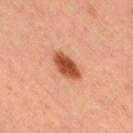Clinical impression: Captured during whole-body skin photography for melanoma surveillance; the lesion was not biopsied. Acquisition and patient details: The total-body-photography lesion software estimated a border-irregularity rating of about 2/10 and radial color variation of about 1. It also reported a classifier nevus-likeness of about 100/100 and a detector confidence of about 100 out of 100 that the crop contains a lesion. The lesion is located on the right thigh. The patient is a female about 35 years old. Captured under cross-polarized illumination. The recorded lesion diameter is about 4.5 mm. A 15 mm close-up tile from a total-body photography series done for melanoma screening.